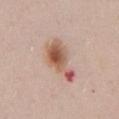Q: Was this lesion biopsied?
A: total-body-photography surveillance lesion; no biopsy
Q: Illumination type?
A: white-light illumination
Q: Where on the body is the lesion?
A: the front of the torso
Q: What is the imaging modality?
A: ~15 mm tile from a whole-body skin photo
Q: What is the lesion's diameter?
A: ≈6 mm
Q: Patient demographics?
A: male, roughly 30 years of age